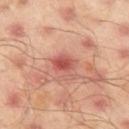Q: Was a biopsy performed?
A: total-body-photography surveillance lesion; no biopsy
Q: What is the anatomic site?
A: the left thigh
Q: Patient demographics?
A: male, about 45 years old
Q: What is the imaging modality?
A: 15 mm crop, total-body photography